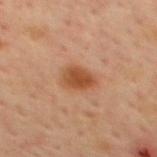The lesion was photographed on a routine skin check and not biopsied; there is no pathology result. From the mid back. About 3.5 mm across. A 15 mm close-up extracted from a 3D total-body photography capture. Imaged with cross-polarized lighting. The total-body-photography lesion software estimated an average lesion color of about L≈49 a*≈25 b*≈36 (CIELAB) and a lesion–skin lightness drop of about 11. The analysis additionally found a border-irregularity index near 2/10, a within-lesion color-variation index near 3.5/10, and radial color variation of about 1.5. A male subject approximately 65 years of age.